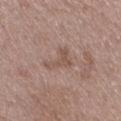Impression: No biopsy was performed on this lesion — it was imaged during a full skin examination and was not determined to be concerning. Background: This is a white-light tile. Cropped from a whole-body photographic skin survey; the tile spans about 15 mm. Located on the right thigh. About 4 mm across. A male subject about 50 years old. Automated tile analysis of the lesion measured an area of roughly 5 mm², an outline eccentricity of about 0.85 (0 = round, 1 = elongated), and a symmetry-axis asymmetry near 0.75. It also reported a border-irregularity rating of about 8/10 and internal color variation of about 1 on a 0–10 scale. The software also gave a classifier nevus-likeness of about 0/100 and a lesion-detection confidence of about 100/100.